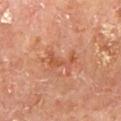| key | value |
|---|---|
| follow-up | no biopsy performed (imaged during a skin exam) |
| lesion size | ~4 mm (longest diameter) |
| subject | male, about 65 years old |
| image source | 15 mm crop, total-body photography |
| tile lighting | cross-polarized illumination |
| site | the back |